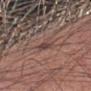Q: Was a biopsy performed?
A: total-body-photography surveillance lesion; no biopsy
Q: Lesion size?
A: about 2.5 mm
Q: Automated lesion metrics?
A: a lesion color around L≈44 a*≈18 b*≈21 in CIELAB, a lesion–skin lightness drop of about 7, and a normalized border contrast of about 6; a border-irregularity index near 3.5/10 and peripheral color asymmetry of about 1; a nevus-likeness score of about 0/100 and a lesion-detection confidence of about 95/100
Q: What lighting was used for the tile?
A: white-light illumination
Q: Lesion location?
A: the arm
Q: Patient demographics?
A: male, approximately 25 years of age
Q: How was this image acquired?
A: ~15 mm tile from a whole-body skin photo